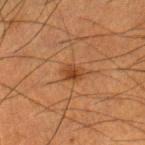An algorithmic analysis of the crop reported a footprint of about 4.5 mm², an outline eccentricity of about 0.65 (0 = round, 1 = elongated), and a shape-asymmetry score of about 0.2 (0 = symmetric). It also reported a lesion–skin lightness drop of about 8 and a lesion-to-skin contrast of about 7 (normalized; higher = more distinct). From the right lower leg. Captured under cross-polarized illumination. A male patient, aged approximately 50. A region of skin cropped from a whole-body photographic capture, roughly 15 mm wide.